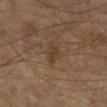The lesion is located on the right lower leg.
A lesion tile, about 15 mm wide, cut from a 3D total-body photograph.
The tile uses cross-polarized illumination.
Longest diameter approximately 2.5 mm.
The subject is aged approximately 55.